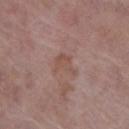Q: Was a biopsy performed?
A: catalogued during a skin exam; not biopsied
Q: What are the patient's age and sex?
A: female, in their 70s
Q: What is the imaging modality?
A: ~15 mm crop, total-body skin-cancer survey
Q: What is the lesion's diameter?
A: about 3 mm
Q: Automated lesion metrics?
A: a border-irregularity index near 5/10, a color-variation rating of about 2/10, and a peripheral color-asymmetry measure near 0.5; a classifier nevus-likeness of about 0/100 and a detector confidence of about 100 out of 100 that the crop contains a lesion
Q: How was the tile lit?
A: white-light illumination
Q: Where on the body is the lesion?
A: the leg